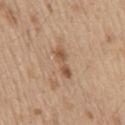Impression: Recorded during total-body skin imaging; not selected for excision or biopsy. Background: Captured under white-light illumination. From the back. A male subject about 70 years old. This image is a 15 mm lesion crop taken from a total-body photograph. Automated tile analysis of the lesion measured a lesion area of about 4.5 mm². The software also gave about 10 CIELAB-L* units darker than the surrounding skin. The software also gave internal color variation of about 2 on a 0–10 scale and radial color variation of about 0.5. Longest diameter approximately 4 mm.